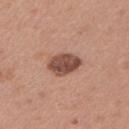notes = total-body-photography surveillance lesion; no biopsy | automated lesion analysis = a footprint of about 9 mm², a shape eccentricity near 0.65, and two-axis asymmetry of about 0.2; internal color variation of about 4 on a 0–10 scale | imaging modality = ~15 mm crop, total-body skin-cancer survey | patient = female, in their 40s | site = the left upper arm | diameter = about 4 mm.